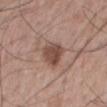{
  "biopsy_status": "not biopsied; imaged during a skin examination",
  "image": {
    "source": "total-body photography crop",
    "field_of_view_mm": 15
  },
  "site": "mid back",
  "lesion_size": {
    "long_diameter_mm_approx": 3.5
  },
  "patient": {
    "sex": "male",
    "age_approx": 70
  },
  "lighting": "white-light"
}